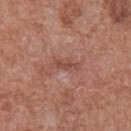notes: no biopsy performed (imaged during a skin exam)
tile lighting: white-light illumination
site: the abdomen
automated metrics: an average lesion color of about L≈47 a*≈25 b*≈28 (CIELAB); a classifier nevus-likeness of about 0/100 and lesion-presence confidence of about 100/100
subject: male, aged 63–67
image source: total-body-photography crop, ~15 mm field of view
diameter: ≈3 mm